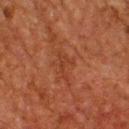  biopsy_status: not biopsied; imaged during a skin examination
  lighting: cross-polarized
  patient:
    sex: male
    age_approx: 60
  site: upper back
  image:
    source: total-body photography crop
    field_of_view_mm: 15